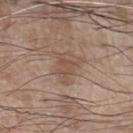Q: Is there a histopathology result?
A: catalogued during a skin exam; not biopsied
Q: Lesion location?
A: the chest
Q: How large is the lesion?
A: about 3.5 mm
Q: How was this image acquired?
A: total-body-photography crop, ~15 mm field of view
Q: Who is the patient?
A: male, in their mid- to late 70s
Q: How was the tile lit?
A: white-light illumination